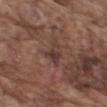The lesion's longest dimension is about 3 mm.
A male patient, roughly 75 years of age.
A close-up tile cropped from a whole-body skin photograph, about 15 mm across.
On the right upper arm.